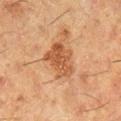No biopsy was performed on this lesion — it was imaged during a full skin examination and was not determined to be concerning.
The total-body-photography lesion software estimated an area of roughly 12 mm², a shape eccentricity near 0.65, and two-axis asymmetry of about 0.25. The analysis additionally found lesion-presence confidence of about 100/100.
A female patient aged around 50.
About 4.5 mm across.
A 15 mm close-up tile from a total-body photography series done for melanoma screening.
Located on the right lower leg.